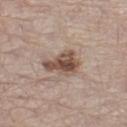  biopsy_status: not biopsied; imaged during a skin examination
  lesion_size:
    long_diameter_mm_approx: 4.5
  image:
    source: total-body photography crop
    field_of_view_mm: 15
  patient:
    sex: male
    age_approx: 65
  site: left thigh